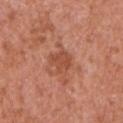* notes: total-body-photography surveillance lesion; no biopsy
* TBP lesion metrics: an average lesion color of about L≈50 a*≈27 b*≈33 (CIELAB), a lesion–skin lightness drop of about 8, and a normalized lesion–skin contrast near 6; border irregularity of about 3 on a 0–10 scale, a color-variation rating of about 2.5/10, and a peripheral color-asymmetry measure near 1; an automated nevus-likeness rating near 0 out of 100 and a lesion-detection confidence of about 100/100
* illumination: white-light
* imaging modality: ~15 mm tile from a whole-body skin photo
* subject: male, in their mid-60s
* lesion size: ≈3 mm
* body site: the left upper arm